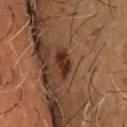biopsy_status: not biopsied; imaged during a skin examination
lighting: cross-polarized
lesion_size:
  long_diameter_mm_approx: 3.5
image:
  source: total-body photography crop
  field_of_view_mm: 15
automated_metrics:
  area_mm2_approx: 5.0
  shape_asymmetry: 0.3
  cielab_L: 29
  cielab_a: 21
  cielab_b: 26
  vs_skin_darker_L: 11.0
  nevus_likeness_0_100: 100
  lesion_detection_confidence_0_100: 100
site: head or neck
patient:
  sex: male
  age_approx: 65